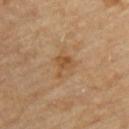Recorded during total-body skin imaging; not selected for excision or biopsy. The patient is a male approximately 85 years of age or older. Captured under cross-polarized illumination. A region of skin cropped from a whole-body photographic capture, roughly 15 mm wide. From the upper back.